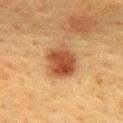The lesion was tiled from a total-body skin photograph and was not biopsied. A female patient, roughly 55 years of age. Located on the upper back. This is a cross-polarized tile. Cropped from a total-body skin-imaging series; the visible field is about 15 mm.